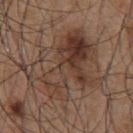image — total-body-photography crop, ~15 mm field of view
TBP lesion metrics — a lesion area of about 37 mm², an outline eccentricity of about 0.7 (0 = round, 1 = elongated), and two-axis asymmetry of about 0.5; a classifier nevus-likeness of about 0/100 and a detector confidence of about 100 out of 100 that the crop contains a lesion
site — the chest
illumination — white-light
diameter — ≈8.5 mm
subject — male, aged 53 to 57